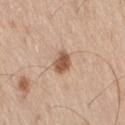patient = male, in their 70s; imaging modality = 15 mm crop, total-body photography; anatomic site = the leg; lesion diameter = ~3 mm (longest diameter); lighting = white-light.